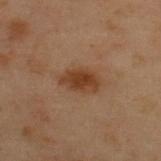The lesion was tiled from a total-body skin photograph and was not biopsied. Measured at roughly 4 mm in maximum diameter. The subject is a male in their mid-50s. A lesion tile, about 15 mm wide, cut from a 3D total-body photograph. Automated image analysis of the tile measured a border-irregularity index near 2.5/10 and peripheral color asymmetry of about 1. It also reported an automated nevus-likeness rating near 90 out of 100. The lesion is on the upper back. The tile uses cross-polarized illumination.